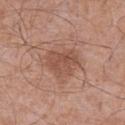{"biopsy_status": "not biopsied; imaged during a skin examination", "site": "abdomen", "patient": {"sex": "male", "age_approx": 70}, "image": {"source": "total-body photography crop", "field_of_view_mm": 15}}